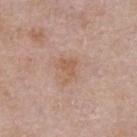• biopsy status: no biopsy performed (imaged during a skin exam)
• diameter: about 3 mm
• anatomic site: the chest
• illumination: white-light illumination
• acquisition: ~15 mm crop, total-body skin-cancer survey
• automated lesion analysis: an area of roughly 4 mm², an outline eccentricity of about 0.7 (0 = round, 1 = elongated), and a symmetry-axis asymmetry near 0.5; an average lesion color of about L≈57 a*≈20 b*≈30 (CIELAB), about 7 CIELAB-L* units darker than the surrounding skin, and a normalized border contrast of about 6; border irregularity of about 5.5 on a 0–10 scale and a within-lesion color-variation index near 1.5/10
• patient: male, aged 53–57